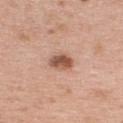<lesion>
<biopsy_status>not biopsied; imaged during a skin examination</biopsy_status>
<automated_metrics>
  <vs_skin_darker_L>15.0</vs_skin_darker_L>
  <vs_skin_contrast_norm>9.5</vs_skin_contrast_norm>
  <border_irregularity_0_10>2.0</border_irregularity_0_10>
  <color_variation_0_10>4.0</color_variation_0_10>
  <peripheral_color_asymmetry>1.5</peripheral_color_asymmetry>
</automated_metrics>
<lighting>white-light</lighting>
<site>upper back</site>
<image>
  <source>total-body photography crop</source>
  <field_of_view_mm>15</field_of_view_mm>
</image>
<patient>
  <sex>male</sex>
  <age_approx>35</age_approx>
</patient>
</lesion>